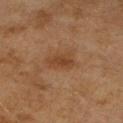The lesion was photographed on a routine skin check and not biopsied; there is no pathology result.
Longest diameter approximately 3.5 mm.
The tile uses cross-polarized illumination.
The lesion is located on the arm.
The subject is a female aged 58–62.
Cropped from a whole-body photographic skin survey; the tile spans about 15 mm.